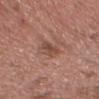Captured during whole-body skin photography for melanoma surveillance; the lesion was not biopsied. On the head or neck. A region of skin cropped from a whole-body photographic capture, roughly 15 mm wide. The total-body-photography lesion software estimated a lesion area of about 5 mm², an outline eccentricity of about 0.85 (0 = round, 1 = elongated), and a symmetry-axis asymmetry near 0.3. The software also gave a lesion–skin lightness drop of about 8 and a lesion-to-skin contrast of about 6.5 (normalized; higher = more distinct). The software also gave a nevus-likeness score of about 0/100. This is a white-light tile. A male subject aged 53 to 57.